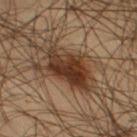Assessment:
Imaged during a routine full-body skin examination; the lesion was not biopsied and no histopathology is available.